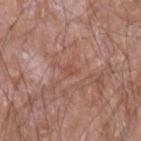Q: Is there a histopathology result?
A: catalogued during a skin exam; not biopsied
Q: What is the imaging modality?
A: ~15 mm crop, total-body skin-cancer survey
Q: What lighting was used for the tile?
A: white-light
Q: What are the patient's age and sex?
A: male, in their 60s
Q: Lesion location?
A: the arm
Q: Lesion size?
A: about 2 mm